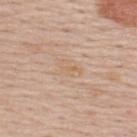| feature | finding |
|---|---|
| biopsy status | total-body-photography surveillance lesion; no biopsy |
| image-analysis metrics | a color-variation rating of about 1.5/10 and a peripheral color-asymmetry measure near 0.5 |
| subject | male, about 60 years old |
| lighting | white-light illumination |
| lesion diameter | about 3 mm |
| acquisition | ~15 mm crop, total-body skin-cancer survey |
| site | the upper back |